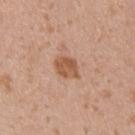Imaged during a routine full-body skin examination; the lesion was not biopsied and no histopathology is available.
A 15 mm crop from a total-body photograph taken for skin-cancer surveillance.
A male patient, approximately 35 years of age.
On the right upper arm.
Measured at roughly 3 mm in maximum diameter.